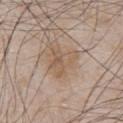The lesion was photographed on a routine skin check and not biopsied; there is no pathology result.
The lesion is on the chest.
Cropped from a total-body skin-imaging series; the visible field is about 15 mm.
A male patient approximately 65 years of age.